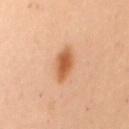Case summary:
– workup — total-body-photography surveillance lesion; no biopsy
– body site — the mid back
– acquisition — 15 mm crop, total-body photography
– patient — female, aged approximately 50
– lighting — cross-polarized
– image-analysis metrics — a mean CIELAB color near L≈50 a*≈22 b*≈34, roughly 12 lightness units darker than nearby skin, and a lesion-to-skin contrast of about 9 (normalized; higher = more distinct); border irregularity of about 2 on a 0–10 scale and a within-lesion color-variation index near 2.5/10; a nevus-likeness score of about 100/100 and a detector confidence of about 100 out of 100 that the crop contains a lesion